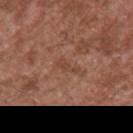- workup · imaged on a skin check; not biopsied
- imaging modality · ~15 mm tile from a whole-body skin photo
- tile lighting · white-light
- location · the back
- patient · male, aged 43 to 47
- TBP lesion metrics · a lesion area of about 2.5 mm², an eccentricity of roughly 0.95, and a symmetry-axis asymmetry near 0.4; a normalized lesion–skin contrast near 5; lesion-presence confidence of about 100/100